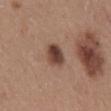Clinical impression: Captured during whole-body skin photography for melanoma surveillance; the lesion was not biopsied. Image and clinical context: Automated tile analysis of the lesion measured an eccentricity of roughly 0.5 and a symmetry-axis asymmetry near 0.2. The software also gave a mean CIELAB color near L≈42 a*≈20 b*≈25, a lesion–skin lightness drop of about 14, and a normalized lesion–skin contrast near 11. The software also gave a classifier nevus-likeness of about 95/100 and a lesion-detection confidence of about 100/100. Cropped from a whole-body photographic skin survey; the tile spans about 15 mm. The lesion is on the mid back. The patient is a male aged 53 to 57. The tile uses white-light illumination. Approximately 3 mm at its widest.